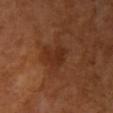The lesion was photographed on a routine skin check and not biopsied; there is no pathology result. The recorded lesion diameter is about 3.5 mm. A female patient aged 53–57. This image is a 15 mm lesion crop taken from a total-body photograph. The lesion is located on the right upper arm. Automated image analysis of the tile measured a lesion area of about 7 mm². The software also gave internal color variation of about 2 on a 0–10 scale and radial color variation of about 0.5. It also reported a classifier nevus-likeness of about 0/100 and lesion-presence confidence of about 100/100.